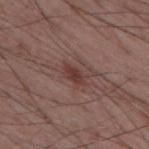Part of a total-body skin-imaging series; this lesion was reviewed on a skin check and was not flagged for biopsy. The recorded lesion diameter is about 3 mm. A male subject, aged around 55. A 15 mm close-up tile from a total-body photography series done for melanoma screening. Located on the back. This is a white-light tile.